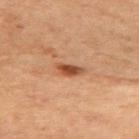<lesion>
  <biopsy_status>not biopsied; imaged during a skin examination</biopsy_status>
  <automated_metrics>
    <area_mm2_approx>3.5</area_mm2_approx>
    <eccentricity>0.9</eccentricity>
    <shape_asymmetry>0.3</shape_asymmetry>
    <vs_skin_darker_L>13.0</vs_skin_darker_L>
    <vs_skin_contrast_norm>10.0</vs_skin_contrast_norm>
    <lesion_detection_confidence_0_100>100</lesion_detection_confidence_0_100>
  </automated_metrics>
  <lesion_size>
    <long_diameter_mm_approx>3.0</long_diameter_mm_approx>
  </lesion_size>
  <site>upper back</site>
  <image>
    <source>total-body photography crop</source>
    <field_of_view_mm>15</field_of_view_mm>
  </image>
  <patient>
    <sex>female</sex>
    <age_approx>60</age_approx>
  </patient>
  <lighting>cross-polarized</lighting>
</lesion>